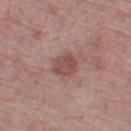notes: catalogued during a skin exam; not biopsied
TBP lesion metrics: a footprint of about 6 mm² and an outline eccentricity of about 0.45 (0 = round, 1 = elongated); a lesion color around L≈49 a*≈22 b*≈23 in CIELAB and about 9 CIELAB-L* units darker than the surrounding skin
location: the left thigh
subject: female, aged around 65
acquisition: ~15 mm crop, total-body skin-cancer survey
tile lighting: white-light
lesion diameter: ≈3 mm